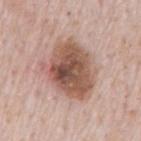Part of a total-body skin-imaging series; this lesion was reviewed on a skin check and was not flagged for biopsy.
Located on the mid back.
The subject is a male aged around 80.
A roughly 15 mm field-of-view crop from a total-body skin photograph.